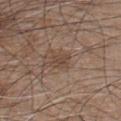workup=no biopsy performed (imaged during a skin exam)
patient=male, aged approximately 45
site=the chest
acquisition=~15 mm tile from a whole-body skin photo